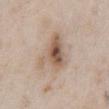Case summary:
* lighting: white-light illumination
* lesion size: ≈6 mm
* image source: total-body-photography crop, ~15 mm field of view
* patient: male, in their mid- to late 60s
* site: the abdomen